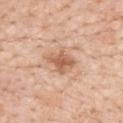Captured during whole-body skin photography for melanoma surveillance; the lesion was not biopsied. An algorithmic analysis of the crop reported an area of roughly 7 mm², a shape eccentricity near 0.7, and a shape-asymmetry score of about 0.25 (0 = symmetric). The analysis additionally found a lesion color around L≈61 a*≈22 b*≈34 in CIELAB, a lesion–skin lightness drop of about 11, and a normalized lesion–skin contrast near 7.5. The software also gave a detector confidence of about 100 out of 100 that the crop contains a lesion. The lesion is located on the back. A female subject, approximately 50 years of age. A 15 mm crop from a total-body photograph taken for skin-cancer surveillance.